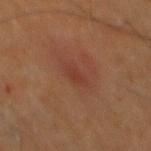workup: total-body-photography surveillance lesion; no biopsy | acquisition: total-body-photography crop, ~15 mm field of view | patient: male, aged around 60 | lesion diameter: ≈3 mm | illumination: cross-polarized | automated metrics: a lesion area of about 3.5 mm² and a symmetry-axis asymmetry near 0.25; an average lesion color of about L≈35 a*≈23 b*≈27 (CIELAB) and a lesion–skin lightness drop of about 5; an automated nevus-likeness rating near 95 out of 100 and lesion-presence confidence of about 100/100 | anatomic site: the back.